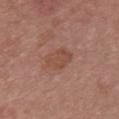Imaged during a routine full-body skin examination; the lesion was not biopsied and no histopathology is available. The recorded lesion diameter is about 3.5 mm. This is a white-light tile. An algorithmic analysis of the crop reported an automated nevus-likeness rating near 5 out of 100 and lesion-presence confidence of about 100/100. The subject is a female roughly 50 years of age. Cropped from a total-body skin-imaging series; the visible field is about 15 mm. Located on the chest.